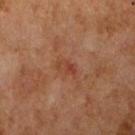Clinical impression:
This lesion was catalogued during total-body skin photography and was not selected for biopsy.
Clinical summary:
The tile uses cross-polarized illumination. Located on the right upper arm. Longest diameter approximately 2.5 mm. A female patient, about 60 years old. Automated image analysis of the tile measured a mean CIELAB color near L≈37 a*≈23 b*≈28, roughly 6 lightness units darker than nearby skin, and a normalized border contrast of about 5.5. The analysis additionally found a border-irregularity index near 3.5/10 and internal color variation of about 1 on a 0–10 scale. And it measured a classifier nevus-likeness of about 0/100 and lesion-presence confidence of about 100/100. Cropped from a total-body skin-imaging series; the visible field is about 15 mm.